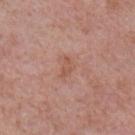Impression:
Captured during whole-body skin photography for melanoma surveillance; the lesion was not biopsied.
Context:
The lesion-visualizer software estimated a lesion–skin lightness drop of about 6 and a normalized lesion–skin contrast near 5.5. And it measured a nevus-likeness score of about 0/100. A male patient, roughly 55 years of age. A lesion tile, about 15 mm wide, cut from a 3D total-body photograph. From the right upper arm.